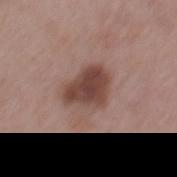<record>
  <biopsy_status>not biopsied; imaged during a skin examination</biopsy_status>
  <lighting>white-light</lighting>
  <patient>
    <sex>male</sex>
    <age_approx>55</age_approx>
  </patient>
  <image>
    <source>total-body photography crop</source>
    <field_of_view_mm>15</field_of_view_mm>
  </image>
  <automated_metrics>
    <area_mm2_approx>12.0</area_mm2_approx>
    <eccentricity>0.6</eccentricity>
    <shape_asymmetry>0.3</shape_asymmetry>
    <nevus_likeness_0_100>40</nevus_likeness_0_100>
  </automated_metrics>
  <lesion_size>
    <long_diameter_mm_approx>4.5</long_diameter_mm_approx>
  </lesion_size>
</record>